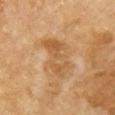{"biopsy_status": "not biopsied; imaged during a skin examination", "automated_metrics": {"cielab_L": 48, "cielab_a": 18, "cielab_b": 35, "vs_skin_darker_L": 7.0, "vs_skin_contrast_norm": 6.0, "border_irregularity_0_10": 5.0, "color_variation_0_10": 4.0, "peripheral_color_asymmetry": 1.0, "nevus_likeness_0_100": 0, "lesion_detection_confidence_0_100": 100}, "site": "front of the torso", "lighting": "cross-polarized", "image": {"source": "total-body photography crop", "field_of_view_mm": 15}, "patient": {"sex": "female", "age_approx": 55}}